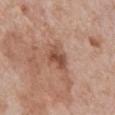Assessment:
The lesion was photographed on a routine skin check and not biopsied; there is no pathology result.
Image and clinical context:
The tile uses white-light illumination. Approximately 3.5 mm at its widest. The subject is a male aged 58–62. A lesion tile, about 15 mm wide, cut from a 3D total-body photograph. The lesion is located on the front of the torso.